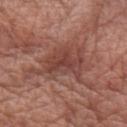Notes:
– workup: total-body-photography surveillance lesion; no biopsy
– illumination: white-light
– acquisition: ~15 mm crop, total-body skin-cancer survey
– body site: the left forearm
– subject: female, in their mid- to late 60s
– lesion diameter: about 7.5 mm